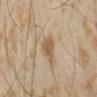tile lighting: cross-polarized
lesion size: ≈3 mm
patient: male, approximately 45 years of age
image source: ~15 mm crop, total-body skin-cancer survey
body site: the arm